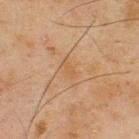• biopsy status — total-body-photography surveillance lesion; no biopsy
• size — about 2.5 mm
• location — the upper back
• subject — male, roughly 45 years of age
• automated metrics — an outline eccentricity of about 0.9 (0 = round, 1 = elongated); a mean CIELAB color near L≈46 a*≈16 b*≈32, about 4 CIELAB-L* units darker than the surrounding skin, and a lesion-to-skin contrast of about 4.5 (normalized; higher = more distinct); a lesion-detection confidence of about 90/100
• tile lighting — cross-polarized
• imaging modality — ~15 mm tile from a whole-body skin photo